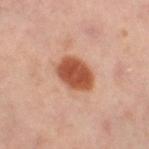Findings:
* biopsy status · catalogued during a skin exam; not biopsied